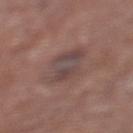A lesion tile, about 15 mm wide, cut from a 3D total-body photograph. Captured under white-light illumination. A male subject, aged 68–72. The total-body-photography lesion software estimated an automated nevus-likeness rating near 0 out of 100 and a lesion-detection confidence of about 100/100. Approximately 5 mm at its widest. The lesion is on the left lower leg.